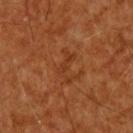<record>
<biopsy_status>not biopsied; imaged during a skin examination</biopsy_status>
<image>
  <source>total-body photography crop</source>
  <field_of_view_mm>15</field_of_view_mm>
</image>
<patient>
  <sex>male</sex>
  <age_approx>60</age_approx>
</patient>
<site>upper back</site>
<lighting>cross-polarized</lighting>
<automated_metrics>
  <area_mm2_approx>5.0</area_mm2_approx>
  <eccentricity>0.9</eccentricity>
  <shape_asymmetry>0.5</shape_asymmetry>
  <color_variation_0_10>0.0</color_variation_0_10>
  <peripheral_color_asymmetry>0.0</peripheral_color_asymmetry>
  <nevus_likeness_0_100>0</nevus_likeness_0_100>
</automated_metrics>
<lesion_size>
  <long_diameter_mm_approx>4.0</long_diameter_mm_approx>
</lesion_size>
</record>